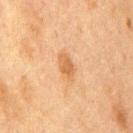Impression: Imaged during a routine full-body skin examination; the lesion was not biopsied and no histopathology is available. Clinical summary: Automated tile analysis of the lesion measured an area of roughly 4.5 mm² and an outline eccentricity of about 0.85 (0 = round, 1 = elongated). The analysis additionally found a classifier nevus-likeness of about 45/100 and a lesion-detection confidence of about 100/100. This is a cross-polarized tile. Located on the chest. A male subject aged around 75. Cropped from a whole-body photographic skin survey; the tile spans about 15 mm. Measured at roughly 3.5 mm in maximum diameter.